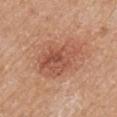{
  "biopsy_status": "not biopsied; imaged during a skin examination",
  "lesion_size": {
    "long_diameter_mm_approx": 6.5
  },
  "lighting": "white-light",
  "automated_metrics": {
    "cielab_L": 54,
    "cielab_a": 25,
    "cielab_b": 32,
    "vs_skin_darker_L": 9.0
  },
  "site": "arm",
  "patient": {
    "sex": "female",
    "age_approx": 75
  },
  "image": {
    "source": "total-body photography crop",
    "field_of_view_mm": 15
  }
}